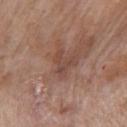The lesion was photographed on a routine skin check and not biopsied; there is no pathology result.